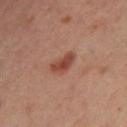{"image": {"source": "total-body photography crop", "field_of_view_mm": 15}, "lesion_size": {"long_diameter_mm_approx": 3.5}, "site": "left leg", "patient": {"sex": "female", "age_approx": 40}}